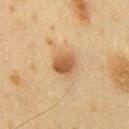Notes:
* workup · catalogued during a skin exam; not biopsied
* body site · the chest
* subject · male, approximately 60 years of age
* acquisition · 15 mm crop, total-body photography